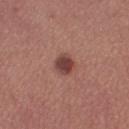Captured during whole-body skin photography for melanoma surveillance; the lesion was not biopsied.
The lesion's longest dimension is about 2.5 mm.
A female subject, aged 28 to 32.
The lesion is on the left lower leg.
Cropped from a whole-body photographic skin survey; the tile spans about 15 mm.
An algorithmic analysis of the crop reported a border-irregularity index near 1/10, internal color variation of about 3 on a 0–10 scale, and a peripheral color-asymmetry measure near 1.
The tile uses white-light illumination.